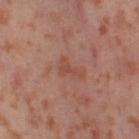Imaged during a routine full-body skin examination; the lesion was not biopsied and no histopathology is available. A female patient aged 53 to 57. An algorithmic analysis of the crop reported a classifier nevus-likeness of about 0/100 and a detector confidence of about 100 out of 100 that the crop contains a lesion. Cropped from a total-body skin-imaging series; the visible field is about 15 mm. The lesion is on the left thigh.